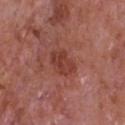Clinical impression: Part of a total-body skin-imaging series; this lesion was reviewed on a skin check and was not flagged for biopsy. Image and clinical context: The subject is a male approximately 65 years of age. Captured under white-light illumination. Located on the chest. Automated image analysis of the tile measured roughly 8 lightness units darker than nearby skin. And it measured a border-irregularity rating of about 2/10, internal color variation of about 3.5 on a 0–10 scale, and a peripheral color-asymmetry measure near 1.5. A close-up tile cropped from a whole-body skin photograph, about 15 mm across. Longest diameter approximately 3.5 mm.